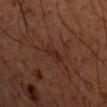Assessment:
This lesion was catalogued during total-body skin photography and was not selected for biopsy.
Image and clinical context:
Approximately 2.5 mm at its widest. A 15 mm close-up tile from a total-body photography series done for melanoma screening. Imaged with cross-polarized lighting. On the left upper arm. A male patient, aged 48 to 52.